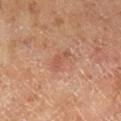Assessment:
Part of a total-body skin-imaging series; this lesion was reviewed on a skin check and was not flagged for biopsy.
Context:
The subject is a male approximately 65 years of age. This is a cross-polarized tile. A close-up tile cropped from a whole-body skin photograph, about 15 mm across. An algorithmic analysis of the crop reported an average lesion color of about L≈53 a*≈24 b*≈31 (CIELAB), about 7 CIELAB-L* units darker than the surrounding skin, and a normalized border contrast of about 5. And it measured a within-lesion color-variation index near 1/10 and peripheral color asymmetry of about 0. It also reported lesion-presence confidence of about 100/100. Longest diameter approximately 3 mm.